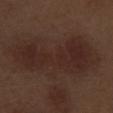No biopsy was performed on this lesion — it was imaged during a full skin examination and was not determined to be concerning. This image is a 15 mm lesion crop taken from a total-body photograph. A male subject aged 68 to 72. The recorded lesion diameter is about 10 mm. On the leg.